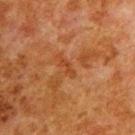Assessment: The lesion was photographed on a routine skin check and not biopsied; there is no pathology result. Context: Imaged with cross-polarized lighting. Automated image analysis of the tile measured a footprint of about 2 mm², an outline eccentricity of about 0.95 (0 = round, 1 = elongated), and a symmetry-axis asymmetry near 0.4. And it measured a border-irregularity rating of about 4.5/10, internal color variation of about 0 on a 0–10 scale, and peripheral color asymmetry of about 0. Cropped from a whole-body photographic skin survey; the tile spans about 15 mm. The lesion's longest dimension is about 2.5 mm. The lesion is on the upper back. A male subject aged 78 to 82.